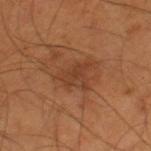notes: total-body-photography surveillance lesion; no biopsy | patient: male, about 55 years old | lesion size: ~3.5 mm (longest diameter) | anatomic site: the leg | lighting: cross-polarized illumination | image: total-body-photography crop, ~15 mm field of view.